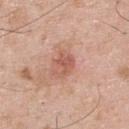Q: Was this lesion biopsied?
A: no biopsy performed (imaged during a skin exam)
Q: How was the tile lit?
A: white-light
Q: What is the anatomic site?
A: the upper back
Q: What is the imaging modality?
A: ~15 mm tile from a whole-body skin photo
Q: What did automated image analysis measure?
A: a lesion color around L≈57 a*≈25 b*≈29 in CIELAB, roughly 9 lightness units darker than nearby skin, and a normalized border contrast of about 6; lesion-presence confidence of about 100/100
Q: Lesion size?
A: about 2.5 mm
Q: What are the patient's age and sex?
A: male, aged around 55